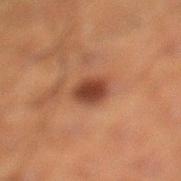{
  "biopsy_status": "not biopsied; imaged during a skin examination"
}